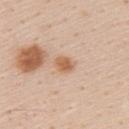Context: The patient is a male in their 60s. Approximately 2.5 mm at its widest. This image is a 15 mm lesion crop taken from a total-body photograph. An algorithmic analysis of the crop reported a lesion color around L≈62 a*≈20 b*≈34 in CIELAB, roughly 10 lightness units darker than nearby skin, and a normalized lesion–skin contrast near 8. And it measured a classifier nevus-likeness of about 40/100 and a lesion-detection confidence of about 100/100. The tile uses white-light illumination. The lesion is on the upper back.